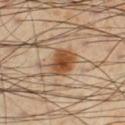workup — catalogued during a skin exam; not biopsied | acquisition — ~15 mm crop, total-body skin-cancer survey | tile lighting — cross-polarized | lesion size — ~4 mm (longest diameter) | patient — male, aged approximately 55 | location — the right lower leg.